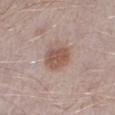anatomic site: the right lower leg | image: ~15 mm crop, total-body skin-cancer survey | patient: male, aged 38–42.